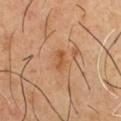  biopsy_status: not biopsied; imaged during a skin examination
  site: front of the torso
  lighting: cross-polarized
  lesion_size:
    long_diameter_mm_approx: 2.5
  image:
    source: total-body photography crop
    field_of_view_mm: 15
  patient:
    sex: male
    age_approx: 55